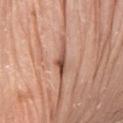| field | value |
|---|---|
| follow-up | imaged on a skin check; not biopsied |
| lesion size | about 3.5 mm |
| acquisition | 15 mm crop, total-body photography |
| subject | male, aged around 80 |
| lighting | white-light |
| anatomic site | the front of the torso |